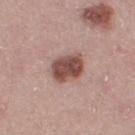The lesion was photographed on a routine skin check and not biopsied; there is no pathology result. The lesion is on the leg. A region of skin cropped from a whole-body photographic capture, roughly 15 mm wide. Captured under white-light illumination. Automated image analysis of the tile measured an eccentricity of roughly 0.75 and a shape-asymmetry score of about 0.15 (0 = symmetric). The software also gave internal color variation of about 4 on a 0–10 scale and peripheral color asymmetry of about 1.5. The subject is a female aged 38 to 42. About 4 mm across.